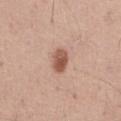About 3 mm across.
A 15 mm crop from a total-body photograph taken for skin-cancer surveillance.
A male subject, aged 53 to 57.
Located on the abdomen.
Automated tile analysis of the lesion measured a mean CIELAB color near L≈55 a*≈22 b*≈28. It also reported a border-irregularity rating of about 1/10, a within-lesion color-variation index near 4/10, and radial color variation of about 1.5. The software also gave a nevus-likeness score of about 95/100.